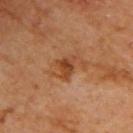{"biopsy_status": "not biopsied; imaged during a skin examination", "lesion_size": {"long_diameter_mm_approx": 3.0}, "site": "upper back", "patient": {"sex": "male", "age_approx": 60}, "automated_metrics": {"border_irregularity_0_10": 4.0, "color_variation_0_10": 4.5, "peripheral_color_asymmetry": 1.5, "nevus_likeness_0_100": 45, "lesion_detection_confidence_0_100": 100}, "lighting": "cross-polarized", "image": {"source": "total-body photography crop", "field_of_view_mm": 15}}